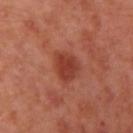notes — catalogued during a skin exam; not biopsied | image-analysis metrics — a lesion area of about 8 mm², an outline eccentricity of about 0.55 (0 = round, 1 = elongated), and two-axis asymmetry of about 0.25; border irregularity of about 2.5 on a 0–10 scale, internal color variation of about 2.5 on a 0–10 scale, and radial color variation of about 1; a classifier nevus-likeness of about 80/100 and a detector confidence of about 100 out of 100 that the crop contains a lesion | location — the right upper arm | patient — female, about 40 years old | lesion size — ~3.5 mm (longest diameter) | illumination — cross-polarized illumination | imaging modality — 15 mm crop, total-body photography.